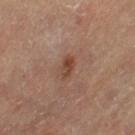Part of a total-body skin-imaging series; this lesion was reviewed on a skin check and was not flagged for biopsy.
A female subject in their mid-60s.
Longest diameter approximately 3 mm.
The tile uses cross-polarized illumination.
The lesion is located on the left thigh.
A lesion tile, about 15 mm wide, cut from a 3D total-body photograph.